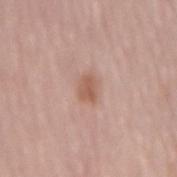{
  "biopsy_status": "not biopsied; imaged during a skin examination",
  "automated_metrics": {
    "area_mm2_approx": 4.5,
    "eccentricity": 0.7,
    "shape_asymmetry": 0.2,
    "cielab_L": 58,
    "cielab_a": 20,
    "cielab_b": 28,
    "vs_skin_contrast_norm": 7.0,
    "border_irregularity_0_10": 2.0,
    "color_variation_0_10": 2.0,
    "peripheral_color_asymmetry": 0.5,
    "nevus_likeness_0_100": 40,
    "lesion_detection_confidence_0_100": 100
  },
  "patient": {
    "sex": "female",
    "age_approx": 65
  },
  "lighting": "white-light",
  "lesion_size": {
    "long_diameter_mm_approx": 3.0
  },
  "image": {
    "source": "total-body photography crop",
    "field_of_view_mm": 15
  },
  "site": "mid back"
}